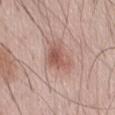notes: catalogued during a skin exam; not biopsied | image: total-body-photography crop, ~15 mm field of view | size: about 4 mm | body site: the front of the torso | tile lighting: white-light illumination | patient: male, in their 60s.